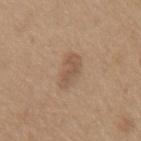biopsy status: no biopsy performed (imaged during a skin exam)
imaging modality: ~15 mm crop, total-body skin-cancer survey
patient: male, in their mid-60s
automated metrics: a mean CIELAB color near L≈54 a*≈16 b*≈30 and a normalized lesion–skin contrast near 5.5; a border-irregularity index near 2.5/10; an automated nevus-likeness rating near 10 out of 100 and a detector confidence of about 100 out of 100 that the crop contains a lesion
lesion diameter: about 4 mm
location: the chest